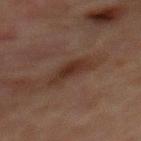Recorded during total-body skin imaging; not selected for excision or biopsy.
The recorded lesion diameter is about 4 mm.
Automated tile analysis of the lesion measured an average lesion color of about L≈24 a*≈15 b*≈20 (CIELAB), about 6 CIELAB-L* units darker than the surrounding skin, and a normalized lesion–skin contrast near 7.5. The software also gave a lesion-detection confidence of about 100/100.
A male subject, aged 63–67.
A lesion tile, about 15 mm wide, cut from a 3D total-body photograph.
The lesion is located on the chest.
The tile uses cross-polarized illumination.